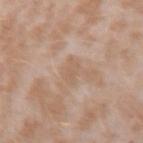{"site": "right forearm", "automated_metrics": {"area_mm2_approx": 3.0, "eccentricity": 0.8, "shape_asymmetry": 0.35, "cielab_L": 59, "cielab_a": 17, "cielab_b": 30, "vs_skin_darker_L": 6.0, "vs_skin_contrast_norm": 4.5, "nevus_likeness_0_100": 0, "lesion_detection_confidence_0_100": 100}, "image": {"source": "total-body photography crop", "field_of_view_mm": 15}, "lesion_size": {"long_diameter_mm_approx": 2.5}, "lighting": "white-light", "patient": {"sex": "female", "age_approx": 25}}